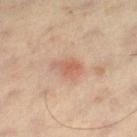notes: imaged on a skin check; not biopsied
acquisition: total-body-photography crop, ~15 mm field of view
automated metrics: a lesion area of about 6 mm², an eccentricity of roughly 0.75, and two-axis asymmetry of about 0.25; a lesion–skin lightness drop of about 8 and a normalized lesion–skin contrast near 6; an automated nevus-likeness rating near 50 out of 100 and lesion-presence confidence of about 100/100
location: the leg
patient: male, in their 60s
size: ~3.5 mm (longest diameter)